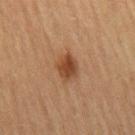Part of a total-body skin-imaging series; this lesion was reviewed on a skin check and was not flagged for biopsy.
A female patient, aged around 80.
On the right thigh.
Imaged with cross-polarized lighting.
The lesion's longest dimension is about 3 mm.
A close-up tile cropped from a whole-body skin photograph, about 15 mm across.